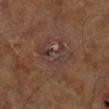notes — no biopsy performed (imaged during a skin exam) | patient — in their mid- to late 60s | TBP lesion metrics — a classifier nevus-likeness of about 0/100 and a detector confidence of about 80 out of 100 that the crop contains a lesion | tile lighting — cross-polarized illumination | acquisition — 15 mm crop, total-body photography | diameter — about 5 mm | location — the right lower leg.